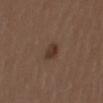The lesion was tiled from a total-body skin photograph and was not biopsied. A female patient, approximately 40 years of age. On the right thigh. This is a white-light tile. The lesion's longest dimension is about 2.5 mm. This image is a 15 mm lesion crop taken from a total-body photograph. The lesion-visualizer software estimated an eccentricity of roughly 0.85 and a shape-asymmetry score of about 0.2 (0 = symmetric). The analysis additionally found about 9 CIELAB-L* units darker than the surrounding skin. The software also gave an automated nevus-likeness rating near 90 out of 100 and lesion-presence confidence of about 100/100.